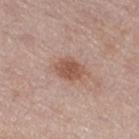{
  "biopsy_status": "not biopsied; imaged during a skin examination",
  "image": {
    "source": "total-body photography crop",
    "field_of_view_mm": 15
  },
  "automated_metrics": {
    "area_mm2_approx": 6.5,
    "eccentricity": 0.7,
    "shape_asymmetry": 0.15,
    "cielab_L": 53,
    "cielab_a": 21,
    "cielab_b": 27,
    "vs_skin_darker_L": 11.0,
    "vs_skin_contrast_norm": 8.0
  },
  "site": "leg",
  "lesion_size": {
    "long_diameter_mm_approx": 3.5
  },
  "patient": {
    "sex": "female",
    "age_approx": 40
  },
  "lighting": "white-light"
}